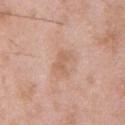workup = catalogued during a skin exam; not biopsied
acquisition = ~15 mm crop, total-body skin-cancer survey
automated metrics = a footprint of about 4.5 mm² and two-axis asymmetry of about 0.35; a lesion color around L≈61 a*≈20 b*≈31 in CIELAB, a lesion–skin lightness drop of about 7, and a normalized lesion–skin contrast near 5.5; a border-irregularity index near 3.5/10, a within-lesion color-variation index near 1.5/10, and peripheral color asymmetry of about 0.5
location = the chest
size = ~3 mm (longest diameter)
patient = male, approximately 50 years of age